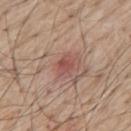The lesion was tiled from a total-body skin photograph and was not biopsied.
The lesion is on the mid back.
This is a white-light tile.
Cropped from a whole-body photographic skin survey; the tile spans about 15 mm.
The subject is a male aged 68 to 72.